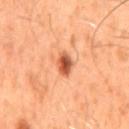Findings:
– notes · no biopsy performed (imaged during a skin exam)
– lesion diameter · ≈3 mm
– body site · the mid back
– image source · total-body-photography crop, ~15 mm field of view
– automated lesion analysis · a border-irregularity index near 2/10 and a within-lesion color-variation index near 5.5/10; a classifier nevus-likeness of about 100/100
– lighting · cross-polarized illumination
– subject · male, aged around 50